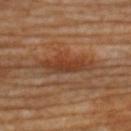Q: Was this lesion biopsied?
A: catalogued during a skin exam; not biopsied
Q: How was the tile lit?
A: cross-polarized
Q: Lesion location?
A: the upper back
Q: Who is the patient?
A: female, aged 63 to 67
Q: Lesion size?
A: about 6.5 mm
Q: What is the imaging modality?
A: 15 mm crop, total-body photography